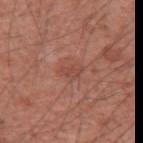biopsy_status: not biopsied; imaged during a skin examination
lesion_size:
  long_diameter_mm_approx: 3.0
automated_metrics:
  area_mm2_approx: 4.0
  eccentricity: 0.8
  shape_asymmetry: 0.35
image:
  source: total-body photography crop
  field_of_view_mm: 15
site: left upper arm
lighting: white-light
patient:
  sex: male
  age_approx: 60